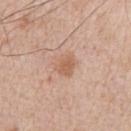Impression: No biopsy was performed on this lesion — it was imaged during a full skin examination and was not determined to be concerning. Background: This image is a 15 mm lesion crop taken from a total-body photograph. The lesion's longest dimension is about 2.5 mm. A male patient, aged 63 to 67. On the right upper arm. The tile uses white-light illumination.